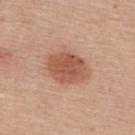{"biopsy_status": "not biopsied; imaged during a skin examination", "lighting": "white-light", "site": "upper back", "lesion_size": {"long_diameter_mm_approx": 5.0}, "automated_metrics": {"cielab_L": 55, "cielab_a": 24, "cielab_b": 31, "vs_skin_darker_L": 12.0, "vs_skin_contrast_norm": 8.0, "border_irregularity_0_10": 1.5, "color_variation_0_10": 3.0, "peripheral_color_asymmetry": 1.0, "lesion_detection_confidence_0_100": 100}, "patient": {"sex": "male", "age_approx": 55}, "image": {"source": "total-body photography crop", "field_of_view_mm": 15}}